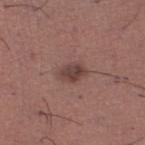<case>
<biopsy_status>not biopsied; imaged during a skin examination</biopsy_status>
<patient>
  <sex>male</sex>
  <age_approx>45</age_approx>
</patient>
<image>
  <source>total-body photography crop</source>
  <field_of_view_mm>15</field_of_view_mm>
</image>
<site>left lower leg</site>
</case>